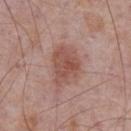<case>
<biopsy_status>not biopsied; imaged during a skin examination</biopsy_status>
<site>chest</site>
<patient>
  <sex>male</sex>
  <age_approx>75</age_approx>
</patient>
<image>
  <source>total-body photography crop</source>
  <field_of_view_mm>15</field_of_view_mm>
</image>
</case>